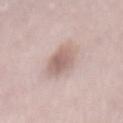Captured during whole-body skin photography for melanoma surveillance; the lesion was not biopsied. A 15 mm close-up tile from a total-body photography series done for melanoma screening. Located on the mid back. A female subject in their 50s. Automated image analysis of the tile measured a footprint of about 9 mm², an outline eccentricity of about 0.9 (0 = round, 1 = elongated), and a shape-asymmetry score of about 0.2 (0 = symmetric). And it measured a mean CIELAB color near L≈62 a*≈17 b*≈22 and a normalized border contrast of about 7. The software also gave lesion-presence confidence of about 85/100.